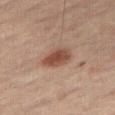follow-up: no biopsy performed (imaged during a skin exam) | lighting: cross-polarized | site: the right thigh | imaging modality: ~15 mm tile from a whole-body skin photo | diameter: ~4 mm (longest diameter) | subject: male, aged 58 to 62.